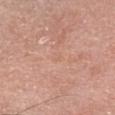Assessment:
No biopsy was performed on this lesion — it was imaged during a full skin examination and was not determined to be concerning.
Acquisition and patient details:
Approximately 1.5 mm at its widest. From the head or neck. A roughly 15 mm field-of-view crop from a total-body skin photograph. A female subject, about 60 years old.